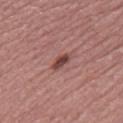<tbp_lesion>
  <biopsy_status>not biopsied; imaged during a skin examination</biopsy_status>
  <image>
    <source>total-body photography crop</source>
    <field_of_view_mm>15</field_of_view_mm>
  </image>
  <site>right lower leg</site>
  <patient>
    <sex>female</sex>
    <age_approx>60</age_approx>
  </patient>
</tbp_lesion>